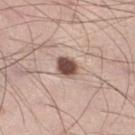follow-up = no biopsy performed (imaged during a skin exam) | patient = male, about 35 years old | location = the right lower leg | acquisition = total-body-photography crop, ~15 mm field of view.